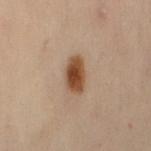Part of a total-body skin-imaging series; this lesion was reviewed on a skin check and was not flagged for biopsy. Longest diameter approximately 4 mm. Captured under cross-polarized illumination. Located on the lower back. A female subject aged 48 to 52. Cropped from a whole-body photographic skin survey; the tile spans about 15 mm.